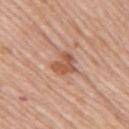No biopsy was performed on this lesion — it was imaged during a full skin examination and was not determined to be concerning. A female subject aged around 65. The lesion is located on the right upper arm. Cropped from a total-body skin-imaging series; the visible field is about 15 mm.